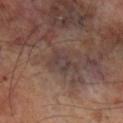Q: Is there a histopathology result?
A: no biopsy performed (imaged during a skin exam)
Q: What did automated image analysis measure?
A: a border-irregularity index near 7.5/10
Q: Lesion location?
A: the right lower leg
Q: Patient demographics?
A: male, aged approximately 70
Q: What is the lesion's diameter?
A: about 3 mm
Q: What kind of image is this?
A: total-body-photography crop, ~15 mm field of view
Q: Illumination type?
A: cross-polarized illumination